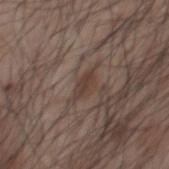The lesion was photographed on a routine skin check and not biopsied; there is no pathology result. Automated image analysis of the tile measured a lesion area of about 3 mm² and an outline eccentricity of about 0.9 (0 = round, 1 = elongated). And it measured a lesion color around L≈38 a*≈15 b*≈22 in CIELAB, a lesion–skin lightness drop of about 8, and a normalized border contrast of about 7. From the chest. A male subject approximately 55 years of age. Captured under white-light illumination. Cropped from a total-body skin-imaging series; the visible field is about 15 mm.